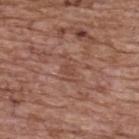Impression:
The lesion was photographed on a routine skin check and not biopsied; there is no pathology result.
Context:
A lesion tile, about 15 mm wide, cut from a 3D total-body photograph. This is a white-light tile. From the back. A female patient, aged around 65.